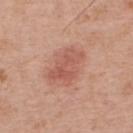Impression: No biopsy was performed on this lesion — it was imaged during a full skin examination and was not determined to be concerning.